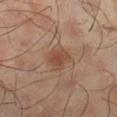Captured during whole-body skin photography for melanoma surveillance; the lesion was not biopsied.
From the right lower leg.
Captured under cross-polarized illumination.
A male patient aged 63–67.
A close-up tile cropped from a whole-body skin photograph, about 15 mm across.
Measured at roughly 3.5 mm in maximum diameter.